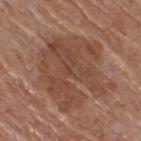The lesion was photographed on a routine skin check and not biopsied; there is no pathology result. The total-body-photography lesion software estimated a shape eccentricity near 0.5 and a shape-asymmetry score of about 0.3 (0 = symmetric). The analysis additionally found an average lesion color of about L≈45 a*≈20 b*≈28 (CIELAB). It also reported a border-irregularity rating of about 6/10, a within-lesion color-variation index near 4/10, and a peripheral color-asymmetry measure near 1.5. And it measured a nevus-likeness score of about 0/100 and lesion-presence confidence of about 100/100. On the right thigh. Captured under white-light illumination. Approximately 8 mm at its widest. A 15 mm close-up extracted from a 3D total-body photography capture. A female patient, approximately 55 years of age.